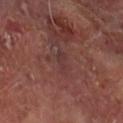| key | value |
|---|---|
| follow-up | no biopsy performed (imaged during a skin exam) |
| anatomic site | the right forearm |
| imaging modality | total-body-photography crop, ~15 mm field of view |
| subject | male, aged 63–67 |
| automated lesion analysis | a lesion-to-skin contrast of about 5.5 (normalized; higher = more distinct); a border-irregularity rating of about 4/10 and a color-variation rating of about 0/10; an automated nevus-likeness rating near 0 out of 100 and a lesion-detection confidence of about 55/100 |
| illumination | cross-polarized illumination |